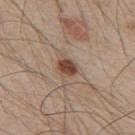| key | value |
|---|---|
| biopsy status | no biopsy performed (imaged during a skin exam) |
| body site | the mid back |
| image source | ~15 mm crop, total-body skin-cancer survey |
| patient | male, aged approximately 50 |